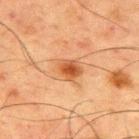notes: total-body-photography surveillance lesion; no biopsy | patient: male, aged 58–62 | image: total-body-photography crop, ~15 mm field of view | site: the upper back | diameter: about 3 mm | illumination: cross-polarized illumination.